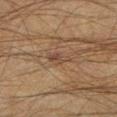No biopsy was performed on this lesion — it was imaged during a full skin examination and was not determined to be concerning. This image is a 15 mm lesion crop taken from a total-body photograph. A male patient, aged approximately 50. Imaged with cross-polarized lighting. About 3 mm across. On the left lower leg.